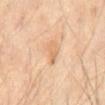follow-up: catalogued during a skin exam; not biopsied
patient: male, about 70 years old
imaging modality: total-body-photography crop, ~15 mm field of view
automated metrics: a mean CIELAB color near L≈67 a*≈20 b*≈37, about 8 CIELAB-L* units darker than the surrounding skin, and a normalized border contrast of about 5.5; a color-variation rating of about 2/10 and radial color variation of about 0.5
size: about 3 mm
illumination: cross-polarized illumination
anatomic site: the abdomen